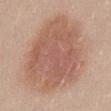Image and clinical context: An algorithmic analysis of the crop reported an average lesion color of about L≈57 a*≈21 b*≈28 (CIELAB), a lesion–skin lightness drop of about 11, and a lesion-to-skin contrast of about 7 (normalized; higher = more distinct). Located on the abdomen. A female subject aged approximately 30. A region of skin cropped from a whole-body photographic capture, roughly 15 mm wide. The tile uses white-light illumination.